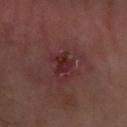Impression:
The lesion was tiled from a total-body skin photograph and was not biopsied.
Context:
The total-body-photography lesion software estimated a lesion color around L≈24 a*≈23 b*≈17 in CIELAB and a normalized lesion–skin contrast near 8. And it measured a nevus-likeness score of about 0/100 and a lesion-detection confidence of about 100/100. A male patient approximately 65 years of age. A 15 mm close-up tile from a total-body photography series done for melanoma screening. Captured under cross-polarized illumination. From the arm.